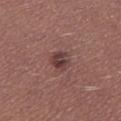tile lighting: white-light illumination | location: the leg | automated lesion analysis: a footprint of about 4.5 mm², an outline eccentricity of about 0.4 (0 = round, 1 = elongated), and a shape-asymmetry score of about 0.25 (0 = symmetric); border irregularity of about 2.5 on a 0–10 scale, a within-lesion color-variation index near 4.5/10, and peripheral color asymmetry of about 1.5 | size: ≈2.5 mm | patient: male, roughly 25 years of age | acquisition: ~15 mm tile from a whole-body skin photo.